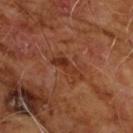<case>
<biopsy_status>not biopsied; imaged during a skin examination</biopsy_status>
</case>